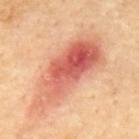• workup — total-body-photography surveillance lesion; no biopsy
• image source — total-body-photography crop, ~15 mm field of view
• size — about 10.5 mm
• subject — male, aged 68 to 72
• anatomic site — the mid back